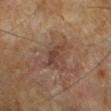The lesion was tiled from a total-body skin photograph and was not biopsied.
The lesion-visualizer software estimated a footprint of about 5 mm², an eccentricity of roughly 0.75, and two-axis asymmetry of about 0.4. The analysis additionally found roughly 7 lightness units darker than nearby skin. The software also gave a border-irregularity rating of about 4/10 and radial color variation of about 0.5. The software also gave a classifier nevus-likeness of about 0/100.
Cropped from a whole-body photographic skin survey; the tile spans about 15 mm.
The tile uses cross-polarized illumination.
The recorded lesion diameter is about 3 mm.
The patient is a male aged around 65.